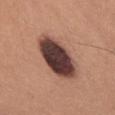{
  "biopsy_status": "not biopsied; imaged during a skin examination",
  "lighting": "white-light",
  "image": {
    "source": "total-body photography crop",
    "field_of_view_mm": 15
  },
  "site": "chest",
  "patient": {
    "sex": "male",
    "age_approx": 35
  }
}